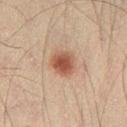TBP lesion metrics: a lesion area of about 7 mm² and an outline eccentricity of about 0.5 (0 = round, 1 = elongated); a classifier nevus-likeness of about 100/100 and lesion-presence confidence of about 100/100
patient: male, about 40 years old
imaging modality: ~15 mm tile from a whole-body skin photo
illumination: cross-polarized
body site: the left thigh
lesion size: ≈3 mm